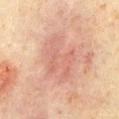Notes:
• body site · the chest
• subject · male, aged approximately 60
• TBP lesion metrics · a lesion area of about 17 mm², an outline eccentricity of about 0.8 (0 = round, 1 = elongated), and two-axis asymmetry of about 0.35; an average lesion color of about L≈53 a*≈20 b*≈25 (CIELAB) and a lesion-to-skin contrast of about 5 (normalized; higher = more distinct); a border-irregularity index near 5.5/10, internal color variation of about 3.5 on a 0–10 scale, and radial color variation of about 1; an automated nevus-likeness rating near 5 out of 100 and a lesion-detection confidence of about 100/100
• image source · total-body-photography crop, ~15 mm field of view